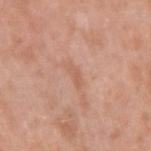Imaged during a routine full-body skin examination; the lesion was not biopsied and no histopathology is available. The tile uses white-light illumination. Located on the right upper arm. Cropped from a whole-body photographic skin survey; the tile spans about 15 mm. A female patient, aged 38 to 42. The recorded lesion diameter is about 3 mm.